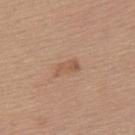Captured during whole-body skin photography for melanoma surveillance; the lesion was not biopsied.
A female patient about 65 years old.
Captured under white-light illumination.
A 15 mm close-up extracted from a 3D total-body photography capture.
The lesion is on the upper back.